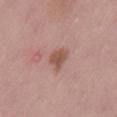notes: imaged on a skin check; not biopsied
subject: male, aged approximately 55
image: 15 mm crop, total-body photography
lighting: white-light illumination
location: the lower back